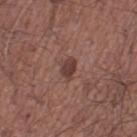Impression: This lesion was catalogued during total-body skin photography and was not selected for biopsy.